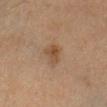Findings:
– workup: total-body-photography surveillance lesion; no biopsy
– image: 15 mm crop, total-body photography
– diameter: ~3 mm (longest diameter)
– illumination: cross-polarized
– patient: female, aged 43 to 47
– anatomic site: the head or neck